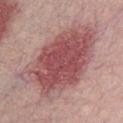Part of a total-body skin-imaging series; this lesion was reviewed on a skin check and was not flagged for biopsy.
The recorded lesion diameter is about 11 mm.
This is a white-light tile.
A 15 mm close-up extracted from a 3D total-body photography capture.
A male patient aged around 30.